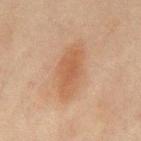Recorded during total-body skin imaging; not selected for excision or biopsy.
The recorded lesion diameter is about 6.5 mm.
This is a cross-polarized tile.
A male patient in their mid- to late 40s.
The lesion is located on the mid back.
A region of skin cropped from a whole-body photographic capture, roughly 15 mm wide.